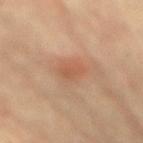Findings:
– notes: no biopsy performed (imaged during a skin exam)
– size: ≈3 mm
– tile lighting: cross-polarized
– image: 15 mm crop, total-body photography
– automated lesion analysis: an eccentricity of roughly 0.8 and a shape-asymmetry score of about 0.25 (0 = symmetric); a nevus-likeness score of about 75/100
– subject: female, roughly 80 years of age
– anatomic site: the mid back